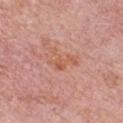Imaged during a routine full-body skin examination; the lesion was not biopsied and no histopathology is available.
A male patient, about 75 years old.
Imaged with white-light lighting.
Cropped from a total-body skin-imaging series; the visible field is about 15 mm.
The lesion is located on the left upper arm.
The lesion's longest dimension is about 3.5 mm.
Automated image analysis of the tile measured a footprint of about 5.5 mm² and a shape eccentricity near 0.8. The software also gave an average lesion color of about L≈58 a*≈25 b*≈33 (CIELAB), about 6 CIELAB-L* units darker than the surrounding skin, and a lesion-to-skin contrast of about 6.5 (normalized; higher = more distinct).